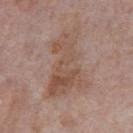{"biopsy_status": "not biopsied; imaged during a skin examination", "site": "chest", "patient": {"sex": "male", "age_approx": 75}, "image": {"source": "total-body photography crop", "field_of_view_mm": 15}}